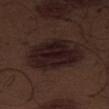Notes:
– notes — total-body-photography surveillance lesion; no biopsy
– image source — ~15 mm crop, total-body skin-cancer survey
– image-analysis metrics — a lesion color around L≈17 a*≈14 b*≈13 in CIELAB and a normalized border contrast of about 13; a border-irregularity index near 2.5/10, internal color variation of about 3.5 on a 0–10 scale, and a peripheral color-asymmetry measure near 1; lesion-presence confidence of about 100/100
– patient — male, aged approximately 70
– lesion size — ≈7.5 mm
– lighting — white-light
– site — the abdomen